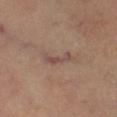biopsy status = no biopsy performed (imaged during a skin exam) | anatomic site = the right lower leg | lesion size = ≈3.5 mm | patient = male, approximately 60 years of age | lighting = cross-polarized | image source = ~15 mm crop, total-body skin-cancer survey.